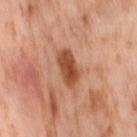Case summary:
– imaging modality · ~15 mm tile from a whole-body skin photo
– body site · the leg
– patient · female, aged 53 to 57
– lighting · cross-polarized illumination